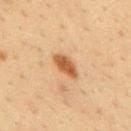The lesion was tiled from a total-body skin photograph and was not biopsied. A male patient, aged around 35. A roughly 15 mm field-of-view crop from a total-body skin photograph. The lesion's longest dimension is about 3.5 mm. Captured under cross-polarized illumination. The lesion is located on the mid back.